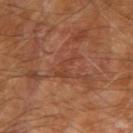biopsy_status: not biopsied; imaged during a skin examination
patient:
  sex: male
  age_approx: 70
lesion_size:
  long_diameter_mm_approx: 3.5
lighting: cross-polarized
site: right upper arm
image:
  source: total-body photography crop
  field_of_view_mm: 15